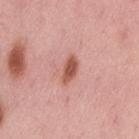biopsy status=no biopsy performed (imaged during a skin exam) | subject=female, in their 50s | automated lesion analysis=border irregularity of about 3 on a 0–10 scale, a within-lesion color-variation index near 2/10, and peripheral color asymmetry of about 0.5 | site=the lower back | acquisition=total-body-photography crop, ~15 mm field of view.